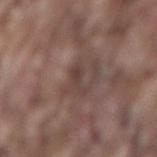  biopsy_status: not biopsied; imaged during a skin examination
  patient:
    sex: male
    age_approx: 75
  site: back
  image:
    source: total-body photography crop
    field_of_view_mm: 15